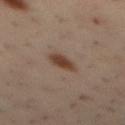Q: Is there a histopathology result?
A: catalogued during a skin exam; not biopsied
Q: Who is the patient?
A: male, approximately 40 years of age
Q: Illumination type?
A: cross-polarized
Q: What is the lesion's diameter?
A: ~3.5 mm (longest diameter)
Q: What kind of image is this?
A: ~15 mm crop, total-body skin-cancer survey
Q: What is the anatomic site?
A: the mid back